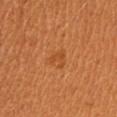Impression: Part of a total-body skin-imaging series; this lesion was reviewed on a skin check and was not flagged for biopsy. Image and clinical context: A female patient aged 28 to 32. The tile uses cross-polarized illumination. The recorded lesion diameter is about 2.5 mm. Located on the right upper arm. This image is a 15 mm lesion crop taken from a total-body photograph.